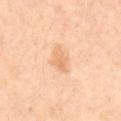The patient is a male aged 68 to 72. A roughly 15 mm field-of-view crop from a total-body skin photograph. From the abdomen. Imaged with cross-polarized lighting.